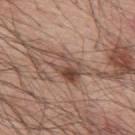  biopsy_status: not biopsied; imaged during a skin examination